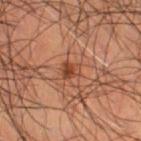workup — catalogued during a skin exam; not biopsied | location — the right thigh | patient — male, in their mid- to late 60s | lighting — cross-polarized | automated metrics — a border-irregularity index near 2.5/10 and peripheral color asymmetry of about 2.5 | size — about 2 mm | image source — ~15 mm crop, total-body skin-cancer survey.